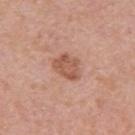Case summary:
• follow-up: no biopsy performed (imaged during a skin exam)
• size: ~3.5 mm (longest diameter)
• imaging modality: ~15 mm tile from a whole-body skin photo
• tile lighting: white-light
• patient: female, about 40 years old
• body site: the left upper arm